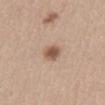Captured during whole-body skin photography for melanoma surveillance; the lesion was not biopsied. About 2.5 mm across. On the front of the torso. A female subject, approximately 40 years of age. A 15 mm crop from a total-body photograph taken for skin-cancer surveillance. The tile uses white-light illumination. The lesion-visualizer software estimated a lesion color around L≈54 a*≈19 b*≈30 in CIELAB, a lesion–skin lightness drop of about 13, and a normalized lesion–skin contrast near 9. The analysis additionally found a within-lesion color-variation index near 2.5/10 and peripheral color asymmetry of about 1.